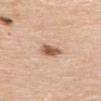Assessment: This lesion was catalogued during total-body skin photography and was not selected for biopsy. Clinical summary: A close-up tile cropped from a whole-body skin photograph, about 15 mm across. About 2.5 mm across. The tile uses white-light illumination. Located on the upper back. A female patient approximately 65 years of age.